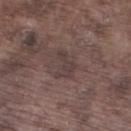notes: imaged on a skin check; not biopsied
acquisition: ~15 mm crop, total-body skin-cancer survey
diameter: ~3.5 mm (longest diameter)
tile lighting: white-light illumination
body site: the leg
automated lesion analysis: an average lesion color of about L≈38 a*≈14 b*≈16 (CIELAB), roughly 6 lightness units darker than nearby skin, and a lesion-to-skin contrast of about 5.5 (normalized; higher = more distinct); a nevus-likeness score of about 0/100 and lesion-presence confidence of about 85/100
subject: male, approximately 75 years of age